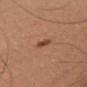notes=total-body-photography surveillance lesion; no biopsy
imaging modality=15 mm crop, total-body photography
body site=the right upper arm
image-analysis metrics=a mean CIELAB color near L≈33 a*≈19 b*≈24 and a normalized lesion–skin contrast near 8
patient=male, about 60 years old
size=~2.5 mm (longest diameter)